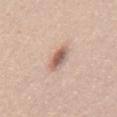workup — total-body-photography surveillance lesion; no biopsy | image source — ~15 mm crop, total-body skin-cancer survey | subject — male, aged around 45 | body site — the back | illumination — white-light.